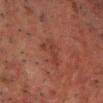The lesion is located on the chest. A region of skin cropped from a whole-body photographic capture, roughly 15 mm wide. Automated image analysis of the tile measured a lesion area of about 5 mm², an outline eccentricity of about 0.85 (0 = round, 1 = elongated), and a symmetry-axis asymmetry near 0.6. A male subject in their 50s.